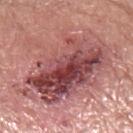| field | value |
|---|---|
| biopsy status | catalogued during a skin exam; not biopsied |
| subject | male, aged approximately 70 |
| acquisition | ~15 mm tile from a whole-body skin photo |
| size | ~13 mm (longest diameter) |
| tile lighting | white-light illumination |
| body site | the arm |